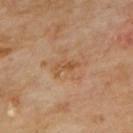| field | value |
|---|---|
| notes | catalogued during a skin exam; not biopsied |
| subject | male, approximately 70 years of age |
| site | the upper back |
| acquisition | ~15 mm crop, total-body skin-cancer survey |